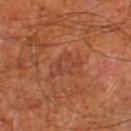follow-up: total-body-photography surveillance lesion; no biopsy
subject: male, aged approximately 65
image: ~15 mm tile from a whole-body skin photo
anatomic site: the left thigh